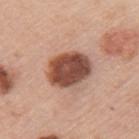Impression: Imaged during a routine full-body skin examination; the lesion was not biopsied and no histopathology is available. Image and clinical context: This is a white-light tile. A region of skin cropped from a whole-body photographic capture, roughly 15 mm wide. From the left upper arm. Approximately 5 mm at its widest. Automated image analysis of the tile measured a footprint of about 16 mm², an outline eccentricity of about 0.55 (0 = round, 1 = elongated), and two-axis asymmetry of about 0.1. The software also gave border irregularity of about 1 on a 0–10 scale, internal color variation of about 5 on a 0–10 scale, and a peripheral color-asymmetry measure near 1.5. A female patient, in their 60s.